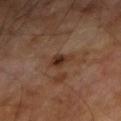Findings:
- workup — no biopsy performed (imaged during a skin exam)
- tile lighting — cross-polarized illumination
- anatomic site — the arm
- patient — male, approximately 70 years of age
- image-analysis metrics — a border-irregularity index near 2.5/10, internal color variation of about 4 on a 0–10 scale, and a peripheral color-asymmetry measure near 1.5; a lesion-detection confidence of about 100/100
- imaging modality — 15 mm crop, total-body photography